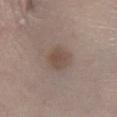Notes:
* workup · total-body-photography surveillance lesion; no biopsy
* imaging modality · total-body-photography crop, ~15 mm field of view
* size · about 3 mm
* location · the leg
* patient · male, aged around 60